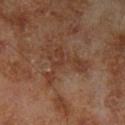Assessment:
Captured during whole-body skin photography for melanoma surveillance; the lesion was not biopsied.
Background:
The lesion is located on the left lower leg. The lesion's longest dimension is about 5.5 mm. The subject is a male aged 68–72. The lesion-visualizer software estimated a normalized border contrast of about 6. Cropped from a whole-body photographic skin survey; the tile spans about 15 mm.